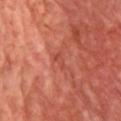Assessment: The lesion was tiled from a total-body skin photograph and was not biopsied. Acquisition and patient details: The patient is a male aged around 65. Measured at roughly 2.5 mm in maximum diameter. On the chest. A 15 mm crop from a total-body photograph taken for skin-cancer surveillance.